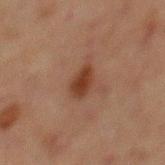biopsy_status: not biopsied; imaged during a skin examination
lesion_size:
  long_diameter_mm_approx: 3.5
lighting: cross-polarized
automated_metrics:
  area_mm2_approx: 5.5
  shape_asymmetry: 0.2
  cielab_L: 31
  cielab_a: 19
  cielab_b: 26
  vs_skin_darker_L: 9.0
  vs_skin_contrast_norm: 9.5
  border_irregularity_0_10: 2.5
  color_variation_0_10: 2.5
  nevus_likeness_0_100: 90
  lesion_detection_confidence_0_100: 100
site: abdomen
image:
  source: total-body photography crop
  field_of_view_mm: 15
patient:
  sex: male
  age_approx: 65